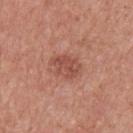workup=total-body-photography surveillance lesion; no biopsy | lesion size=≈3.5 mm | image source=total-body-photography crop, ~15 mm field of view | patient=male, aged around 65 | site=the upper back | tile lighting=white-light illumination.